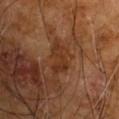Case summary:
- follow-up · no biopsy performed (imaged during a skin exam)
- image source · ~15 mm tile from a whole-body skin photo
- diameter · ≈4.5 mm
- body site · the right arm
- image-analysis metrics · an area of roughly 6 mm², a shape eccentricity near 0.9, and a shape-asymmetry score of about 0.35 (0 = symmetric); border irregularity of about 5 on a 0–10 scale and peripheral color asymmetry of about 1; lesion-presence confidence of about 95/100
- subject · male, aged approximately 60
- illumination · cross-polarized illumination